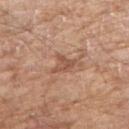Q: Was a biopsy performed?
A: no biopsy performed (imaged during a skin exam)